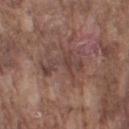Impression:
The lesion was tiled from a total-body skin photograph and was not biopsied.
Clinical summary:
The lesion is on the right upper arm. This is a white-light tile. A male subject aged 73 to 77. This image is a 15 mm lesion crop taken from a total-body photograph.